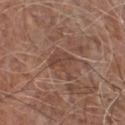The lesion's longest dimension is about 4 mm. Captured under white-light illumination. Located on the chest. A 15 mm close-up tile from a total-body photography series done for melanoma screening. An algorithmic analysis of the crop reported a footprint of about 7 mm², an outline eccentricity of about 0.65 (0 = round, 1 = elongated), and a symmetry-axis asymmetry near 0.5. The analysis additionally found an automated nevus-likeness rating near 0 out of 100 and lesion-presence confidence of about 55/100. The patient is a male approximately 80 years of age.